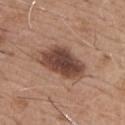  biopsy_status: not biopsied; imaged during a skin examination
  image:
    source: total-body photography crop
    field_of_view_mm: 15
  automated_metrics:
    eccentricity: 0.85
    shape_asymmetry: 0.25
    border_irregularity_0_10: 2.5
    color_variation_0_10: 5.0
  lesion_size:
    long_diameter_mm_approx: 6.0
  lighting: white-light
  patient:
    sex: male
    age_approx: 65
  site: chest